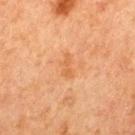This lesion was catalogued during total-body skin photography and was not selected for biopsy.